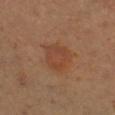Q: Was this lesion biopsied?
A: imaged on a skin check; not biopsied
Q: Illumination type?
A: cross-polarized
Q: Who is the patient?
A: female, aged 43 to 47
Q: Where on the body is the lesion?
A: the left leg
Q: What is the imaging modality?
A: total-body-photography crop, ~15 mm field of view